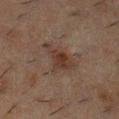  biopsy_status: not biopsied; imaged during a skin examination
  image:
    source: total-body photography crop
    field_of_view_mm: 15
  patient:
    sex: male
    age_approx: 50
  lesion_size:
    long_diameter_mm_approx: 4.5
  site: chest
  automated_metrics:
    area_mm2_approx: 7.0
    eccentricity: 0.85
    shape_asymmetry: 0.5